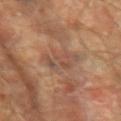Q: Is there a histopathology result?
A: imaged on a skin check; not biopsied
Q: Lesion location?
A: the left upper arm
Q: What is the imaging modality?
A: total-body-photography crop, ~15 mm field of view
Q: Patient demographics?
A: male, aged around 65
Q: How was the tile lit?
A: cross-polarized illumination
Q: How large is the lesion?
A: ≈4 mm